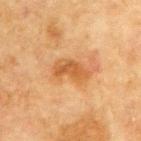subject: male, in their mid-60s
image source: ~15 mm crop, total-body skin-cancer survey
lesion size: ~4.5 mm (longest diameter)
TBP lesion metrics: a lesion area of about 8 mm²; internal color variation of about 2.5 on a 0–10 scale and a peripheral color-asymmetry measure near 1; a classifier nevus-likeness of about 5/100
tile lighting: cross-polarized illumination
location: the chest